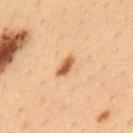follow-up — catalogued during a skin exam; not biopsied
patient — male, aged around 35
acquisition — 15 mm crop, total-body photography
lighting — cross-polarized illumination
site — the upper back